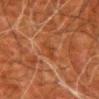Impression: The lesion was photographed on a routine skin check and not biopsied; there is no pathology result. Background: On the left upper arm. The subject is a male aged approximately 80. This is a cross-polarized tile. An algorithmic analysis of the crop reported an outline eccentricity of about 0.9 (0 = round, 1 = elongated). And it measured a classifier nevus-likeness of about 0/100. The recorded lesion diameter is about 2.5 mm. Cropped from a total-body skin-imaging series; the visible field is about 15 mm.